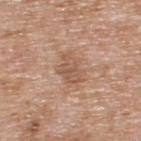Recorded during total-body skin imaging; not selected for excision or biopsy. Captured under white-light illumination. The recorded lesion diameter is about 4 mm. A 15 mm crop from a total-body photograph taken for skin-cancer surveillance. The patient is a male approximately 60 years of age. The lesion is on the upper back.